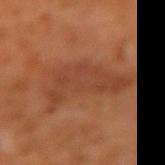The lesion was tiled from a total-body skin photograph and was not biopsied. A male patient approximately 60 years of age. A region of skin cropped from a whole-body photographic capture, roughly 15 mm wide. From the left forearm.